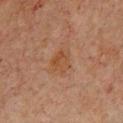notes: imaged on a skin check; not biopsied | acquisition: total-body-photography crop, ~15 mm field of view | lighting: cross-polarized | location: the chest | patient: male, roughly 65 years of age | lesion diameter: ≈3 mm.